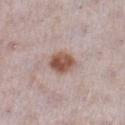Captured during whole-body skin photography for melanoma surveillance; the lesion was not biopsied.
The subject is a female in their 30s.
The lesion's longest dimension is about 3.5 mm.
This image is a 15 mm lesion crop taken from a total-body photograph.
Located on the right lower leg.